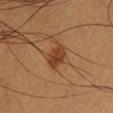Assessment: No biopsy was performed on this lesion — it was imaged during a full skin examination and was not determined to be concerning. Background: From the chest. A male patient, aged approximately 50. Longest diameter approximately 3 mm. A roughly 15 mm field-of-view crop from a total-body skin photograph. Automated image analysis of the tile measured a lesion color around L≈31 a*≈19 b*≈27 in CIELAB, a lesion–skin lightness drop of about 9, and a normalized border contrast of about 8.5. Captured under cross-polarized illumination.